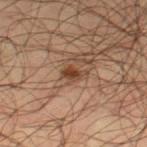Q: Is there a histopathology result?
A: catalogued during a skin exam; not biopsied
Q: Who is the patient?
A: male, aged 33 to 37
Q: Where on the body is the lesion?
A: the right thigh
Q: How large is the lesion?
A: about 2.5 mm
Q: Automated lesion metrics?
A: a lesion area of about 3 mm², an eccentricity of roughly 0.8, and a shape-asymmetry score of about 0.4 (0 = symmetric); a classifier nevus-likeness of about 85/100
Q: How was this image acquired?
A: 15 mm crop, total-body photography